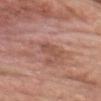Captured during whole-body skin photography for melanoma surveillance; the lesion was not biopsied. The lesion's longest dimension is about 3 mm. A region of skin cropped from a whole-body photographic capture, roughly 15 mm wide. Captured under white-light illumination. A male patient aged 58–62. On the front of the torso.